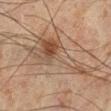{
  "biopsy_status": "not biopsied; imaged during a skin examination",
  "lesion_size": {
    "long_diameter_mm_approx": 11.5
  },
  "patient": {
    "sex": "male",
    "age_approx": 45
  },
  "site": "left lower leg",
  "lighting": "cross-polarized",
  "image": {
    "source": "total-body photography crop",
    "field_of_view_mm": 15
  }
}